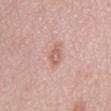{
  "biopsy_status": "not biopsied; imaged during a skin examination",
  "image": {
    "source": "total-body photography crop",
    "field_of_view_mm": 15
  },
  "site": "mid back",
  "lesion_size": {
    "long_diameter_mm_approx": 3.0
  },
  "patient": {
    "sex": "female",
    "age_approx": 70
  },
  "lighting": "white-light"
}